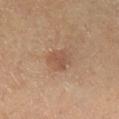Q: Was this lesion biopsied?
A: total-body-photography surveillance lesion; no biopsy
Q: What lighting was used for the tile?
A: cross-polarized illumination
Q: What is the lesion's diameter?
A: ≈2.5 mm
Q: Patient demographics?
A: female, aged 58–62
Q: What kind of image is this?
A: 15 mm crop, total-body photography
Q: Lesion location?
A: the right lower leg